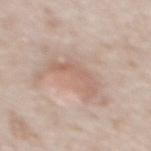Notes:
- subject · female, roughly 40 years of age
- size · ≈4.5 mm
- acquisition · 15 mm crop, total-body photography
- site · the upper back
- lighting · white-light illumination